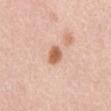Impression:
The lesion was photographed on a routine skin check and not biopsied; there is no pathology result.
Clinical summary:
On the front of the torso. The total-body-photography lesion software estimated a lesion area of about 4 mm², an outline eccentricity of about 0.7 (0 = round, 1 = elongated), and two-axis asymmetry of about 0.2. Approximately 2.5 mm at its widest. A 15 mm crop from a total-body photograph taken for skin-cancer surveillance. A male subject, aged approximately 50.